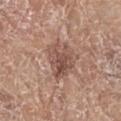<case>
<image>
  <source>total-body photography crop</source>
  <field_of_view_mm>15</field_of_view_mm>
</image>
<site>left lower leg</site>
<patient>
  <sex>female</sex>
  <age_approx>75</age_approx>
</patient>
<lighting>white-light</lighting>
</case>